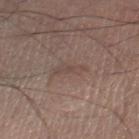| field | value |
|---|---|
| workup | no biopsy performed (imaged during a skin exam) |
| illumination | white-light |
| body site | the right thigh |
| imaging modality | ~15 mm crop, total-body skin-cancer survey |
| subject | male, aged around 55 |
| automated lesion analysis | a footprint of about 4 mm² and an outline eccentricity of about 0.95 (0 = round, 1 = elongated); a lesion color around L≈46 a*≈15 b*≈22 in CIELAB, about 6 CIELAB-L* units darker than the surrounding skin, and a normalized border contrast of about 4.5; a nevus-likeness score of about 0/100 and lesion-presence confidence of about 90/100 |
| size | ≈4 mm |